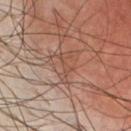Part of a total-body skin-imaging series; this lesion was reviewed on a skin check and was not flagged for biopsy. The lesion-visualizer software estimated a lesion area of about 3.5 mm², a shape eccentricity near 0.95, and a shape-asymmetry score of about 0.5 (0 = symmetric). It also reported an average lesion color of about L≈47 a*≈20 b*≈27 (CIELAB) and a lesion–skin lightness drop of about 6. The software also gave a classifier nevus-likeness of about 0/100 and a detector confidence of about 75 out of 100 that the crop contains a lesion. Cropped from a whole-body photographic skin survey; the tile spans about 15 mm. The lesion is located on the chest. Captured under cross-polarized illumination. The patient is a male about 35 years old.